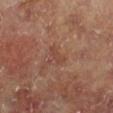Clinical summary:
The lesion is on the right lower leg. A region of skin cropped from a whole-body photographic capture, roughly 15 mm wide. The subject is a male in their 70s. Longest diameter approximately 2.5 mm.